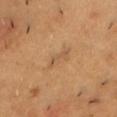| feature | finding |
|---|---|
| biopsy status | catalogued during a skin exam; not biopsied |
| automated lesion analysis | an area of roughly 3.5 mm²; a lesion–skin lightness drop of about 6 and a normalized lesion–skin contrast near 4.5; border irregularity of about 5.5 on a 0–10 scale, a within-lesion color-variation index near 0/10, and peripheral color asymmetry of about 0; a classifier nevus-likeness of about 0/100 and lesion-presence confidence of about 95/100 |
| size | about 3.5 mm |
| subject | male, approximately 45 years of age |
| site | the chest |
| imaging modality | total-body-photography crop, ~15 mm field of view |
| lighting | cross-polarized |